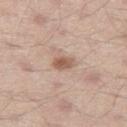Q: Is there a histopathology result?
A: no biopsy performed (imaged during a skin exam)
Q: Lesion size?
A: about 2.5 mm
Q: What lighting was used for the tile?
A: white-light
Q: Patient demographics?
A: male, aged around 35
Q: Lesion location?
A: the left thigh
Q: What is the imaging modality?
A: ~15 mm tile from a whole-body skin photo